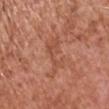Assessment:
Captured during whole-body skin photography for melanoma surveillance; the lesion was not biopsied.
Image and clinical context:
Located on the chest. Imaged with white-light lighting. A male subject in their mid-70s. A 15 mm close-up extracted from a 3D total-body photography capture. Automated image analysis of the tile measured a lesion area of about 7 mm² and a symmetry-axis asymmetry near 0.4. It also reported a mean CIELAB color near L≈51 a*≈25 b*≈32, roughly 6 lightness units darker than nearby skin, and a normalized lesion–skin contrast near 5. The analysis additionally found border irregularity of about 7 on a 0–10 scale, a within-lesion color-variation index near 1.5/10, and peripheral color asymmetry of about 0.5. It also reported a classifier nevus-likeness of about 0/100 and a lesion-detection confidence of about 100/100.